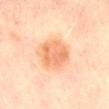Q: Is there a histopathology result?
A: imaged on a skin check; not biopsied
Q: Illumination type?
A: cross-polarized
Q: Where on the body is the lesion?
A: the chest
Q: What kind of image is this?
A: total-body-photography crop, ~15 mm field of view
Q: What are the patient's age and sex?
A: female, aged around 50
Q: How large is the lesion?
A: ~5 mm (longest diameter)